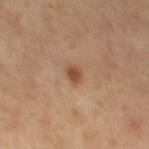Imaged during a routine full-body skin examination; the lesion was not biopsied and no histopathology is available. Cropped from a whole-body photographic skin survey; the tile spans about 15 mm. The subject is a female about 65 years old. Located on the left thigh.